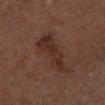No biopsy was performed on this lesion — it was imaged during a full skin examination and was not determined to be concerning.
A male subject approximately 75 years of age.
The tile uses white-light illumination.
Located on the head or neck.
A roughly 15 mm field-of-view crop from a total-body skin photograph.
Approximately 7 mm at its widest.